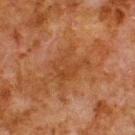{"biopsy_status": "not biopsied; imaged during a skin examination", "patient": {"sex": "male", "age_approx": 80}, "site": "upper back", "lesion_size": {"long_diameter_mm_approx": 4.5}, "image": {"source": "total-body photography crop", "field_of_view_mm": 15}}